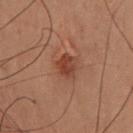Part of a total-body skin-imaging series; this lesion was reviewed on a skin check and was not flagged for biopsy. A roughly 15 mm field-of-view crop from a total-body skin photograph. The lesion's longest dimension is about 3.5 mm. On the front of the torso. A male patient about 60 years old.